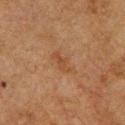This lesion was catalogued during total-body skin photography and was not selected for biopsy. A male subject roughly 75 years of age. The lesion's longest dimension is about 4 mm. A roughly 15 mm field-of-view crop from a total-body skin photograph. The lesion-visualizer software estimated an outline eccentricity of about 0.85 (0 = round, 1 = elongated). The lesion is located on the chest.